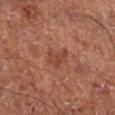Assessment: The lesion was photographed on a routine skin check and not biopsied; there is no pathology result. Background: A patient aged 63 to 67. The lesion is on the right lower leg. The lesion's longest dimension is about 3 mm. A region of skin cropped from a whole-body photographic capture, roughly 15 mm wide. Imaged with cross-polarized lighting. The total-body-photography lesion software estimated a lesion area of about 4 mm² and a symmetry-axis asymmetry near 0.5. And it measured a mean CIELAB color near L≈43 a*≈25 b*≈30, a lesion–skin lightness drop of about 7, and a normalized lesion–skin contrast near 6.